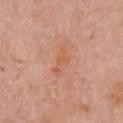Imaged during a routine full-body skin examination; the lesion was not biopsied and no histopathology is available.
A female patient roughly 65 years of age.
The recorded lesion diameter is about 3.5 mm.
Automated image analysis of the tile measured roughly 6 lightness units darker than nearby skin. And it measured a within-lesion color-variation index near 0.5/10 and a peripheral color-asymmetry measure near 0. And it measured a detector confidence of about 100 out of 100 that the crop contains a lesion.
A close-up tile cropped from a whole-body skin photograph, about 15 mm across.
The tile uses white-light illumination.
From the chest.